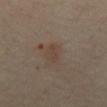Captured during whole-body skin photography for melanoma surveillance; the lesion was not biopsied.
A 15 mm close-up extracted from a 3D total-body photography capture.
A female subject, approximately 40 years of age.
The lesion is on the abdomen.
Imaged with cross-polarized lighting.
The lesion-visualizer software estimated an area of roughly 3 mm² and a symmetry-axis asymmetry near 0.4. And it measured about 5 CIELAB-L* units darker than the surrounding skin and a lesion-to-skin contrast of about 5 (normalized; higher = more distinct). The software also gave a border-irregularity index near 4.5/10 and peripheral color asymmetry of about 0.